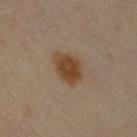{"biopsy_status": "not biopsied; imaged during a skin examination", "patient": {"sex": "female", "age_approx": 45}, "site": "right upper arm", "lesion_size": {"long_diameter_mm_approx": 4.0}, "image": {"source": "total-body photography crop", "field_of_view_mm": 15}, "lighting": "cross-polarized", "automated_metrics": {"area_mm2_approx": 9.0, "shape_asymmetry": 0.2, "border_irregularity_0_10": 2.0, "color_variation_0_10": 3.0, "nevus_likeness_0_100": 100, "lesion_detection_confidence_0_100": 100}}